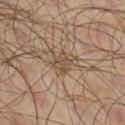No biopsy was performed on this lesion — it was imaged during a full skin examination and was not determined to be concerning. A male subject, about 65 years old. The lesion's longest dimension is about 3 mm. A 15 mm crop from a total-body photograph taken for skin-cancer surveillance. On the right lower leg.